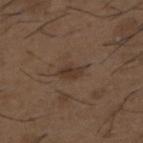workup = catalogued during a skin exam; not biopsied
image-analysis metrics = an area of roughly 4 mm², a shape eccentricity near 0.85, and two-axis asymmetry of about 0.3; an automated nevus-likeness rating near 0 out of 100 and a lesion-detection confidence of about 100/100
anatomic site = the upper back
imaging modality = ~15 mm crop, total-body skin-cancer survey
patient = male, roughly 50 years of age
diameter = ~3 mm (longest diameter)